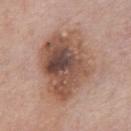Recorded during total-body skin imaging; not selected for excision or biopsy.